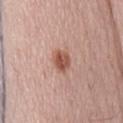Impression: This lesion was catalogued during total-body skin photography and was not selected for biopsy. Acquisition and patient details: Located on the mid back. A male subject, aged around 65. A roughly 15 mm field-of-view crop from a total-body skin photograph.